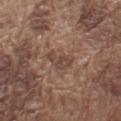Findings:
– workup — imaged on a skin check; not biopsied
– site — the left upper arm
– lighting — white-light
– automated metrics — a lesion area of about 5 mm², an outline eccentricity of about 0.85 (0 = round, 1 = elongated), and a symmetry-axis asymmetry near 0.4; a mean CIELAB color near L≈44 a*≈17 b*≈24, a lesion–skin lightness drop of about 7, and a lesion-to-skin contrast of about 6 (normalized; higher = more distinct)
– lesion size — about 3.5 mm
– imaging modality — ~15 mm tile from a whole-body skin photo
– patient — male, roughly 75 years of age